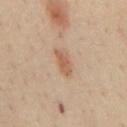Part of a total-body skin-imaging series; this lesion was reviewed on a skin check and was not flagged for biopsy.
A 15 mm crop from a total-body photograph taken for skin-cancer surveillance.
The subject is a male aged around 45.
The lesion is on the mid back.